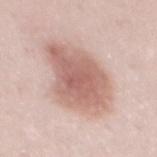The lesion was tiled from a total-body skin photograph and was not biopsied.
From the mid back.
A male subject aged approximately 25.
A 15 mm close-up extracted from a 3D total-body photography capture.